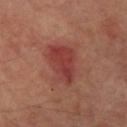Impression: The lesion was tiled from a total-body skin photograph and was not biopsied. Image and clinical context: Captured under cross-polarized illumination. A 15 mm close-up extracted from a 3D total-body photography capture. Measured at roughly 5 mm in maximum diameter. A female patient, in their mid-60s. Located on the right forearm.